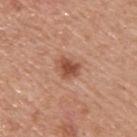Clinical impression: This lesion was catalogued during total-body skin photography and was not selected for biopsy. Context: From the upper back. A close-up tile cropped from a whole-body skin photograph, about 15 mm across. This is a white-light tile. A male subject approximately 55 years of age.